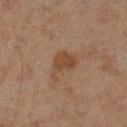imaging modality: total-body-photography crop, ~15 mm field of view
anatomic site: the leg
subject: male, in their mid- to late 40s
lesion diameter: about 3.5 mm